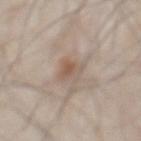follow-up: imaged on a skin check; not biopsied | anatomic site: the chest | imaging modality: total-body-photography crop, ~15 mm field of view | patient: male, roughly 55 years of age.